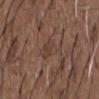The lesion was tiled from a total-body skin photograph and was not biopsied.